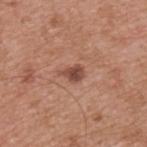Assessment:
This lesion was catalogued during total-body skin photography and was not selected for biopsy.
Context:
The subject is a male aged around 55. A 15 mm close-up extracted from a 3D total-body photography capture. The tile uses white-light illumination. Approximately 2.5 mm at its widest. On the back.